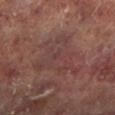Clinical impression:
The lesion was photographed on a routine skin check and not biopsied; there is no pathology result.
Clinical summary:
A region of skin cropped from a whole-body photographic capture, roughly 15 mm wide. This is a cross-polarized tile. The lesion is located on the left lower leg. A male patient, aged 63 to 67.